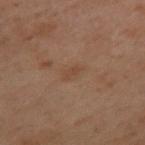biopsy_status: not biopsied; imaged during a skin examination
patient:
  sex: female
  age_approx: 55
image:
  source: total-body photography crop
  field_of_view_mm: 15
site: upper back